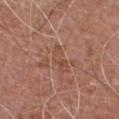Imaged during a routine full-body skin examination; the lesion was not biopsied and no histopathology is available. Cropped from a whole-body photographic skin survey; the tile spans about 15 mm. Imaged with white-light lighting. Automated image analysis of the tile measured a lesion area of about 5 mm², an outline eccentricity of about 0.9 (0 = round, 1 = elongated), and two-axis asymmetry of about 0.4. It also reported an average lesion color of about L≈49 a*≈21 b*≈30 (CIELAB), roughly 5 lightness units darker than nearby skin, and a normalized border contrast of about 5. And it measured an automated nevus-likeness rating near 0 out of 100 and lesion-presence confidence of about 70/100. Longest diameter approximately 4 mm. A male subject approximately 55 years of age. On the chest.